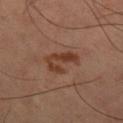Part of a total-body skin-imaging series; this lesion was reviewed on a skin check and was not flagged for biopsy. On the right thigh. Measured at roughly 4.5 mm in maximum diameter. A male patient, in their 60s. Captured under cross-polarized illumination. A 15 mm close-up extracted from a 3D total-body photography capture.